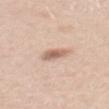biopsy status: total-body-photography surveillance lesion; no biopsy
location: the mid back
automated lesion analysis: an average lesion color of about L≈63 a*≈18 b*≈28 (CIELAB) and a lesion-to-skin contrast of about 7.5 (normalized; higher = more distinct)
acquisition: ~15 mm tile from a whole-body skin photo
patient: female, aged 38–42